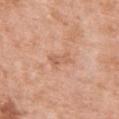Background: On the front of the torso. The patient is a female about 50 years old. Automated image analysis of the tile measured border irregularity of about 3 on a 0–10 scale, a color-variation rating of about 2/10, and peripheral color asymmetry of about 0.5. The analysis additionally found a detector confidence of about 100 out of 100 that the crop contains a lesion. Captured under white-light illumination. A close-up tile cropped from a whole-body skin photograph, about 15 mm across.